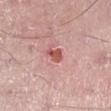Clinical impression: Captured during whole-body skin photography for melanoma surveillance; the lesion was not biopsied. Acquisition and patient details: Approximately 2.5 mm at its widest. The patient is a male aged 48–52. The lesion is located on the right lower leg. A 15 mm crop from a total-body photograph taken for skin-cancer surveillance. Captured under white-light illumination.